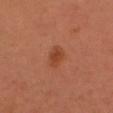The lesion was tiled from a total-body skin photograph and was not biopsied.
A female patient, aged around 50.
This is a cross-polarized tile.
Approximately 3 mm at its widest.
The lesion-visualizer software estimated a footprint of about 4.5 mm², a shape eccentricity near 0.75, and two-axis asymmetry of about 0.25. It also reported a normalized border contrast of about 6.5.
A region of skin cropped from a whole-body photographic capture, roughly 15 mm wide.